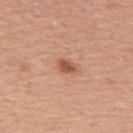Q: Was this lesion biopsied?
A: total-body-photography surveillance lesion; no biopsy
Q: What lighting was used for the tile?
A: white-light
Q: What is the lesion's diameter?
A: about 2.5 mm
Q: Lesion location?
A: the upper back
Q: Patient demographics?
A: female, roughly 60 years of age
Q: What is the imaging modality?
A: total-body-photography crop, ~15 mm field of view
Q: Automated lesion metrics?
A: border irregularity of about 1.5 on a 0–10 scale, a color-variation rating of about 3/10, and peripheral color asymmetry of about 1